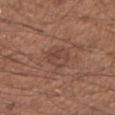Imaged during a routine full-body skin examination; the lesion was not biopsied and no histopathology is available. A 15 mm close-up extracted from a 3D total-body photography capture. This is a white-light tile. Automated tile analysis of the lesion measured an outline eccentricity of about 0.5 (0 = round, 1 = elongated). The analysis additionally found internal color variation of about 4 on a 0–10 scale and a peripheral color-asymmetry measure near 1.5. And it measured an automated nevus-likeness rating near 0 out of 100 and a detector confidence of about 100 out of 100 that the crop contains a lesion. The subject is a male aged 48 to 52. The lesion is located on the right upper arm.